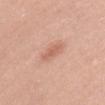  biopsy_status: not biopsied; imaged during a skin examination
  site: abdomen
  patient:
    sex: female
    age_approx: 50
  lesion_size:
    long_diameter_mm_approx: 3.0
  image:
    source: total-body photography crop
    field_of_view_mm: 15